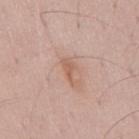The lesion was photographed on a routine skin check and not biopsied; there is no pathology result.
Automated tile analysis of the lesion measured an area of roughly 3 mm², an outline eccentricity of about 0.9 (0 = round, 1 = elongated), and two-axis asymmetry of about 0.4. The analysis additionally found an average lesion color of about L≈59 a*≈20 b*≈30 (CIELAB), about 8 CIELAB-L* units darker than the surrounding skin, and a normalized border contrast of about 6.5. The software also gave a border-irregularity rating of about 4.5/10, a color-variation rating of about 0.5/10, and radial color variation of about 0. The analysis additionally found lesion-presence confidence of about 100/100.
Measured at roughly 3 mm in maximum diameter.
On the back.
The subject is a male aged approximately 50.
This is a white-light tile.
A region of skin cropped from a whole-body photographic capture, roughly 15 mm wide.